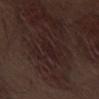Case summary:
* notes: imaged on a skin check; not biopsied
* lesion size: about 2.5 mm
* patient: male, aged approximately 70
* acquisition: 15 mm crop, total-body photography
* TBP lesion metrics: a lesion area of about 3 mm², an eccentricity of roughly 0.85, and a symmetry-axis asymmetry near 0.3; border irregularity of about 3 on a 0–10 scale and peripheral color asymmetry of about 0; a classifier nevus-likeness of about 0/100 and a lesion-detection confidence of about 95/100
* anatomic site: the leg